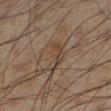Impression: Captured during whole-body skin photography for melanoma surveillance; the lesion was not biopsied. Context: A region of skin cropped from a whole-body photographic capture, roughly 15 mm wide. This is a cross-polarized tile. The patient is a male aged 53–57. The lesion is on the left lower leg.